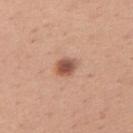follow-up: imaged on a skin check; not biopsied
patient: female, aged 28 to 32
acquisition: total-body-photography crop, ~15 mm field of view
diameter: ≈3 mm
lighting: white-light illumination
automated lesion analysis: a lesion color around L≈54 a*≈23 b*≈30 in CIELAB, a lesion–skin lightness drop of about 14, and a normalized lesion–skin contrast near 9; a within-lesion color-variation index near 6/10 and peripheral color asymmetry of about 2
location: the left upper arm